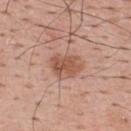Q: Is there a histopathology result?
A: imaged on a skin check; not biopsied
Q: What is the anatomic site?
A: the upper back
Q: How was this image acquired?
A: ~15 mm tile from a whole-body skin photo
Q: Who is the patient?
A: male, approximately 65 years of age
Q: How large is the lesion?
A: about 4 mm
Q: Automated lesion metrics?
A: an area of roughly 9.5 mm², an eccentricity of roughly 0.7, and a symmetry-axis asymmetry near 0.3; a lesion–skin lightness drop of about 10; border irregularity of about 2.5 on a 0–10 scale and radial color variation of about 1.5; a nevus-likeness score of about 45/100 and a detector confidence of about 100 out of 100 that the crop contains a lesion